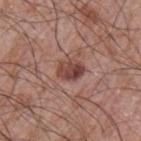The lesion was tiled from a total-body skin photograph and was not biopsied.
The lesion is located on the left upper arm.
A male patient, about 65 years old.
Longest diameter approximately 3 mm.
This is a white-light tile.
A 15 mm crop from a total-body photograph taken for skin-cancer surveillance.